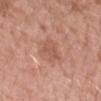Impression: Imaged during a routine full-body skin examination; the lesion was not biopsied and no histopathology is available. Context: Imaged with white-light lighting. From the right forearm. A male subject, aged approximately 60. A 15 mm crop from a total-body photograph taken for skin-cancer surveillance.